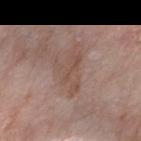biopsy status: catalogued during a skin exam; not biopsied
site: the arm
size: about 5 mm
automated lesion analysis: a lesion–skin lightness drop of about 7 and a lesion-to-skin contrast of about 5.5 (normalized; higher = more distinct); a border-irregularity index near 8.5/10, internal color variation of about 2.5 on a 0–10 scale, and a peripheral color-asymmetry measure near 1; a nevus-likeness score of about 0/100 and lesion-presence confidence of about 100/100
subject: female, aged around 65
image source: total-body-photography crop, ~15 mm field of view